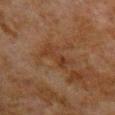Impression:
Imaged during a routine full-body skin examination; the lesion was not biopsied and no histopathology is available.
Clinical summary:
Approximately 4 mm at its widest. A 15 mm close-up extracted from a 3D total-body photography capture. This is a cross-polarized tile. A male patient, aged 78 to 82. Located on the left upper arm.